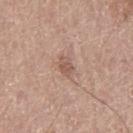| key | value |
|---|---|
| notes | catalogued during a skin exam; not biopsied |
| location | the left thigh |
| patient | male, aged around 65 |
| acquisition | ~15 mm crop, total-body skin-cancer survey |
| lesion size | ~3 mm (longest diameter) |
| lighting | white-light |